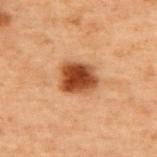This lesion was catalogued during total-body skin photography and was not selected for biopsy.
A 15 mm close-up tile from a total-body photography series done for melanoma screening.
On the upper back.
A male patient aged approximately 70.